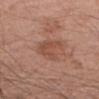This lesion was catalogued during total-body skin photography and was not selected for biopsy. From the right forearm. A roughly 15 mm field-of-view crop from a total-body skin photograph. The lesion-visualizer software estimated a lesion color around L≈49 a*≈23 b*≈28 in CIELAB and a lesion–skin lightness drop of about 8. It also reported a border-irregularity index near 5/10, a within-lesion color-variation index near 2.5/10, and radial color variation of about 1. The software also gave a lesion-detection confidence of about 100/100. A male subject aged 58 to 62.